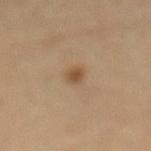<record>
<image>
  <source>total-body photography crop</source>
  <field_of_view_mm>15</field_of_view_mm>
</image>
<site>mid back</site>
<patient>
  <sex>male</sex>
  <age_approx>60</age_approx>
</patient>
<automated_metrics>
  <border_irregularity_0_10>2.0</border_irregularity_0_10>
  <color_variation_0_10>2.5</color_variation_0_10>
  <peripheral_color_asymmetry>0.5</peripheral_color_asymmetry>
  <nevus_likeness_0_100>90</nevus_likeness_0_100>
  <lesion_detection_confidence_0_100>100</lesion_detection_confidence_0_100>
</automated_metrics>
</record>